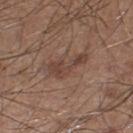Imaged during a routine full-body skin examination; the lesion was not biopsied and no histopathology is available.
A close-up tile cropped from a whole-body skin photograph, about 15 mm across.
The tile uses white-light illumination.
A male patient, aged 58 to 62.
From the right lower leg.